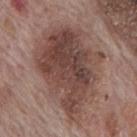Imaged during a routine full-body skin examination; the lesion was not biopsied and no histopathology is available. A 15 mm crop from a total-body photograph taken for skin-cancer surveillance. On the back. A male subject, aged around 70. The recorded lesion diameter is about 10.5 mm. Imaged with white-light lighting.